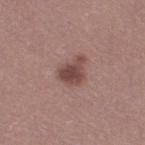Q: Was a biopsy performed?
A: no biopsy performed (imaged during a skin exam)
Q: How was the tile lit?
A: white-light
Q: What kind of image is this?
A: ~15 mm crop, total-body skin-cancer survey
Q: Automated lesion metrics?
A: a mean CIELAB color near L≈45 a*≈20 b*≈21, a lesion–skin lightness drop of about 12, and a normalized lesion–skin contrast near 9
Q: What are the patient's age and sex?
A: female, in their mid-30s
Q: Lesion location?
A: the left leg